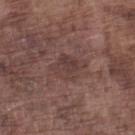Impression: No biopsy was performed on this lesion — it was imaged during a full skin examination and was not determined to be concerning. Image and clinical context: A lesion tile, about 15 mm wide, cut from a 3D total-body photograph. The subject is a male in their mid-70s. The lesion is on the left lower leg. Measured at roughly 4 mm in maximum diameter.